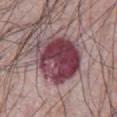Case summary:
– workup — total-body-photography surveillance lesion; no biopsy